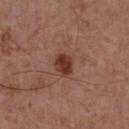A region of skin cropped from a whole-body photographic capture, roughly 15 mm wide.
Longest diameter approximately 2.5 mm.
The lesion is on the front of the torso.
A male subject, roughly 55 years of age.